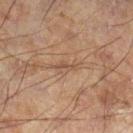image source: 15 mm crop, total-body photography
lesion size: about 2.5 mm
illumination: cross-polarized illumination
TBP lesion metrics: an area of roughly 2.5 mm², an outline eccentricity of about 0.9 (0 = round, 1 = elongated), and a symmetry-axis asymmetry near 0.4; a border-irregularity rating of about 5/10, a within-lesion color-variation index near 0/10, and radial color variation of about 0
body site: the left leg
patient: male, about 60 years old